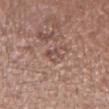notes: no biopsy performed (imaged during a skin exam)
TBP lesion metrics: an eccentricity of roughly 0.85; an average lesion color of about L≈50 a*≈19 b*≈24 (CIELAB) and a lesion-to-skin contrast of about 6 (normalized; higher = more distinct); border irregularity of about 4.5 on a 0–10 scale, a color-variation rating of about 2/10, and a peripheral color-asymmetry measure near 0.5; a nevus-likeness score of about 0/100 and lesion-presence confidence of about 90/100
illumination: white-light illumination
anatomic site: the right forearm
subject: female, about 50 years old
image source: 15 mm crop, total-body photography
size: ≈3 mm